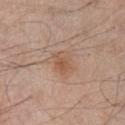Imaged during a routine full-body skin examination; the lesion was not biopsied and no histopathology is available. The lesion-visualizer software estimated a border-irregularity rating of about 2.5/10 and a color-variation rating of about 3.5/10. The software also gave an automated nevus-likeness rating near 70 out of 100 and lesion-presence confidence of about 100/100. This is a white-light tile. Located on the chest. Cropped from a whole-body photographic skin survey; the tile spans about 15 mm. About 3.5 mm across. A male subject, approximately 80 years of age.